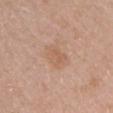Assessment: Imaged during a routine full-body skin examination; the lesion was not biopsied and no histopathology is available. Background: Automated image analysis of the tile measured a lesion area of about 6 mm² and an eccentricity of roughly 0.65. It also reported a border-irregularity index near 2.5/10. The lesion is located on the arm. A close-up tile cropped from a whole-body skin photograph, about 15 mm across. Captured under white-light illumination. The lesion's longest dimension is about 3 mm. A female subject, in their mid- to late 60s.